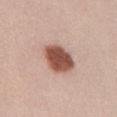| key | value |
|---|---|
| biopsy status | imaged on a skin check; not biopsied |
| anatomic site | the abdomen |
| diameter | ~4.5 mm (longest diameter) |
| imaging modality | total-body-photography crop, ~15 mm field of view |
| lighting | white-light illumination |
| patient | female, in their mid-20s |